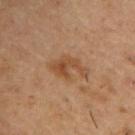  biopsy_status: not biopsied; imaged during a skin examination
  patient:
    sex: male
    age_approx: 55
  site: right upper arm
  lighting: cross-polarized
  image:
    source: total-body photography crop
    field_of_view_mm: 15
  lesion_size:
    long_diameter_mm_approx: 4.5